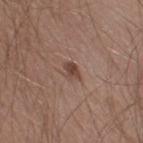The lesion was photographed on a routine skin check and not biopsied; there is no pathology result.
The lesion-visualizer software estimated an area of roughly 3.5 mm², an eccentricity of roughly 0.75, and two-axis asymmetry of about 0.35. The software also gave an average lesion color of about L≈44 a*≈18 b*≈24 (CIELAB) and a normalized lesion–skin contrast near 7.5. And it measured border irregularity of about 3 on a 0–10 scale and radial color variation of about 1. The analysis additionally found a nevus-likeness score of about 85/100 and a detector confidence of about 100 out of 100 that the crop contains a lesion.
Captured under white-light illumination.
The lesion is on the right thigh.
A lesion tile, about 15 mm wide, cut from a 3D total-body photograph.
About 2.5 mm across.
The subject is a male about 30 years old.